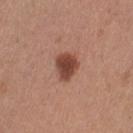Captured during whole-body skin photography for melanoma surveillance; the lesion was not biopsied.
A region of skin cropped from a whole-body photographic capture, roughly 15 mm wide.
This is a white-light tile.
Longest diameter approximately 3.5 mm.
A female subject approximately 30 years of age.
Located on the left thigh.
The total-body-photography lesion software estimated a normalized border contrast of about 10.5. It also reported a nevus-likeness score of about 100/100 and lesion-presence confidence of about 100/100.